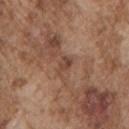No biopsy was performed on this lesion — it was imaged during a full skin examination and was not determined to be concerning. A region of skin cropped from a whole-body photographic capture, roughly 15 mm wide. The patient is a male approximately 75 years of age. Longest diameter approximately 2.5 mm. The lesion is located on the left upper arm.